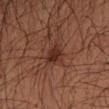* biopsy status: total-body-photography surveillance lesion; no biopsy